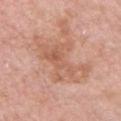Recorded during total-body skin imaging; not selected for excision or biopsy.
From the chest.
A male subject in their mid- to late 50s.
Imaged with white-light lighting.
Automated tile analysis of the lesion measured a border-irregularity rating of about 8/10, a color-variation rating of about 4.5/10, and a peripheral color-asymmetry measure near 1.5. The analysis additionally found a nevus-likeness score of about 0/100 and a detector confidence of about 100 out of 100 that the crop contains a lesion.
A roughly 15 mm field-of-view crop from a total-body skin photograph.